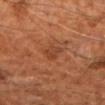Notes:
* biopsy status: no biopsy performed (imaged during a skin exam)
* tile lighting: cross-polarized
* diameter: about 3 mm
* subject: male, roughly 60 years of age
* image source: ~15 mm tile from a whole-body skin photo
* automated lesion analysis: an area of roughly 3 mm², an outline eccentricity of about 0.9 (0 = round, 1 = elongated), and a symmetry-axis asymmetry near 0.3; border irregularity of about 3.5 on a 0–10 scale, a within-lesion color-variation index near 2/10, and peripheral color asymmetry of about 0.5
* anatomic site: the right forearm